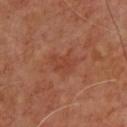acquisition: ~15 mm crop, total-body skin-cancer survey
patient: male, roughly 65 years of age
image-analysis metrics: an outline eccentricity of about 0.7 (0 = round, 1 = elongated); about 6 CIELAB-L* units darker than the surrounding skin and a normalized lesion–skin contrast near 5; an automated nevus-likeness rating near 0 out of 100 and a lesion-detection confidence of about 100/100